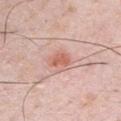Q: Is there a histopathology result?
A: imaged on a skin check; not biopsied
Q: What are the patient's age and sex?
A: male, aged around 35
Q: What lighting was used for the tile?
A: white-light illumination
Q: How large is the lesion?
A: ~2.5 mm (longest diameter)
Q: What is the imaging modality?
A: total-body-photography crop, ~15 mm field of view
Q: Lesion location?
A: the chest